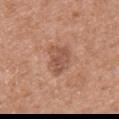Case summary:
- notes · imaged on a skin check; not biopsied